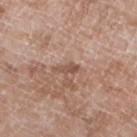Notes:
- workup — no biopsy performed (imaged during a skin exam)
- anatomic site — the leg
- acquisition — ~15 mm crop, total-body skin-cancer survey
- subject — male, aged 58–62